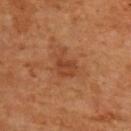• notes: total-body-photography surveillance lesion; no biopsy
• lighting: cross-polarized
• size: about 3.5 mm
• patient: male, aged around 65
• location: the upper back
• imaging modality: total-body-photography crop, ~15 mm field of view